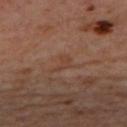subject: female, aged 43–47 | TBP lesion metrics: an area of roughly 2.5 mm², a shape eccentricity near 0.8, and two-axis asymmetry of about 0.55; an average lesion color of about L≈39 a*≈19 b*≈27 (CIELAB) and a lesion-to-skin contrast of about 5 (normalized; higher = more distinct); a within-lesion color-variation index near 1/10 | location: the upper back | acquisition: ~15 mm tile from a whole-body skin photo | tile lighting: cross-polarized.